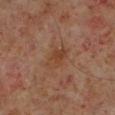Q: Was this lesion biopsied?
A: imaged on a skin check; not biopsied
Q: How was this image acquired?
A: 15 mm crop, total-body photography
Q: Where on the body is the lesion?
A: the left lower leg
Q: What is the lesion's diameter?
A: ≈3.5 mm
Q: Illumination type?
A: cross-polarized illumination
Q: Who is the patient?
A: male, roughly 60 years of age